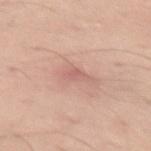Image and clinical context:
The lesion is on the left thigh. A lesion tile, about 15 mm wide, cut from a 3D total-body photograph. The patient is a male aged 48 to 52.